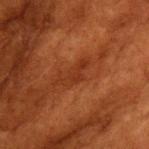<tbp_lesion>
  <biopsy_status>not biopsied; imaged during a skin examination</biopsy_status>
  <lesion_size>
    <long_diameter_mm_approx>4.5</long_diameter_mm_approx>
  </lesion_size>
  <image>
    <source>total-body photography crop</source>
    <field_of_view_mm>15</field_of_view_mm>
  </image>
  <lighting>cross-polarized</lighting>
  <patient>
    <sex>female</sex>
    <age_approx>80</age_approx>
  </patient>
  <site>front of the torso</site>
</tbp_lesion>